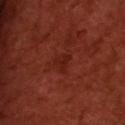Impression:
No biopsy was performed on this lesion — it was imaged during a full skin examination and was not determined to be concerning.